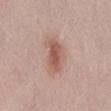workup = imaged on a skin check; not biopsied
subject = male, aged 28 to 32
imaging modality = ~15 mm tile from a whole-body skin photo
tile lighting = white-light